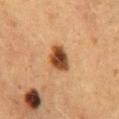notes — imaged on a skin check; not biopsied
anatomic site — the lower back
patient — female, about 50 years old
lighting — cross-polarized illumination
acquisition — ~15 mm tile from a whole-body skin photo